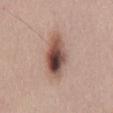notes: no biopsy performed (imaged during a skin exam)
automated lesion analysis: a mean CIELAB color near L≈49 a*≈20 b*≈24, a lesion–skin lightness drop of about 18, and a lesion-to-skin contrast of about 12 (normalized; higher = more distinct); a border-irregularity index near 2.5/10 and a color-variation rating of about 10/10
lighting: white-light illumination
location: the lower back
diameter: ≈6 mm
patient: male, aged 43 to 47
image: ~15 mm tile from a whole-body skin photo